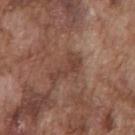Recorded during total-body skin imaging; not selected for excision or biopsy.
Imaged with white-light lighting.
This image is a 15 mm lesion crop taken from a total-body photograph.
Approximately 4 mm at its widest.
From the chest.
A male patient, in their mid-70s.